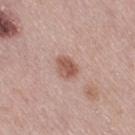No biopsy was performed on this lesion — it was imaged during a full skin examination and was not determined to be concerning.
A region of skin cropped from a whole-body photographic capture, roughly 15 mm wide.
The subject is a female in their mid- to late 60s.
The lesion is located on the right thigh.
The lesion's longest dimension is about 2.5 mm.